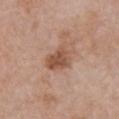Image and clinical context: Captured under white-light illumination. A region of skin cropped from a whole-body photographic capture, roughly 15 mm wide. Located on the chest. Measured at roughly 3.5 mm in maximum diameter. The subject is a female aged approximately 40.